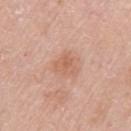biopsy status: no biopsy performed (imaged during a skin exam) | lighting: white-light | patient: male, approximately 80 years of age | imaging modality: 15 mm crop, total-body photography | site: the right upper arm | diameter: ≈2.5 mm.